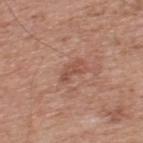Findings:
- workup: total-body-photography surveillance lesion; no biopsy
- acquisition: 15 mm crop, total-body photography
- body site: the upper back
- tile lighting: white-light
- patient: male, aged approximately 55